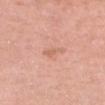The tile uses white-light illumination.
The lesion is on the head or neck.
A female subject, aged around 55.
Cropped from a total-body skin-imaging series; the visible field is about 15 mm.
An algorithmic analysis of the crop reported a footprint of about 2.5 mm² and a shape-asymmetry score of about 0.45 (0 = symmetric). It also reported a mean CIELAB color near L≈64 a*≈24 b*≈31, a lesion–skin lightness drop of about 7, and a normalized border contrast of about 5. The software also gave a border-irregularity rating of about 5.5/10, internal color variation of about 0 on a 0–10 scale, and a peripheral color-asymmetry measure near 0. It also reported a classifier nevus-likeness of about 0/100 and a detector confidence of about 100 out of 100 that the crop contains a lesion.
Longest diameter approximately 3 mm.